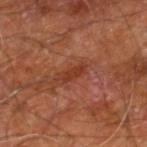No biopsy was performed on this lesion — it was imaged during a full skin examination and was not determined to be concerning.
A 15 mm close-up extracted from a 3D total-body photography capture.
The lesion is on the right leg.
The lesion-visualizer software estimated a within-lesion color-variation index near 0.5/10 and radial color variation of about 0.
A male patient, aged around 60.
Imaged with cross-polarized lighting.
The recorded lesion diameter is about 3 mm.